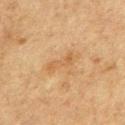A male subject aged 73 to 77. Approximately 4 mm at its widest. This is a cross-polarized tile. A 15 mm close-up tile from a total-body photography series done for melanoma screening. Located on the front of the torso. The lesion-visualizer software estimated an area of roughly 4.5 mm². And it measured a classifier nevus-likeness of about 0/100 and a lesion-detection confidence of about 100/100.